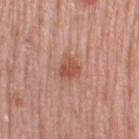Clinical impression:
This lesion was catalogued during total-body skin photography and was not selected for biopsy.
Clinical summary:
Automated tile analysis of the lesion measured border irregularity of about 2.5 on a 0–10 scale and a peripheral color-asymmetry measure near 1. Imaged with white-light lighting. Measured at roughly 3 mm in maximum diameter. The patient is a female roughly 65 years of age. A 15 mm close-up tile from a total-body photography series done for melanoma screening. On the right thigh.